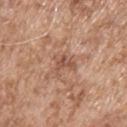Q: Was a biopsy performed?
A: total-body-photography surveillance lesion; no biopsy
Q: What did automated image analysis measure?
A: a footprint of about 4 mm² and two-axis asymmetry of about 0.65; a nevus-likeness score of about 0/100
Q: Lesion location?
A: the upper back
Q: How was the tile lit?
A: white-light
Q: Who is the patient?
A: male, approximately 55 years of age
Q: Lesion size?
A: ≈2.5 mm
Q: What is the imaging modality?
A: ~15 mm crop, total-body skin-cancer survey